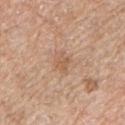biopsy status: total-body-photography surveillance lesion; no biopsy
location: the left upper arm
subject: male, aged 53–57
tile lighting: white-light
image source: ~15 mm crop, total-body skin-cancer survey
lesion diameter: about 3 mm